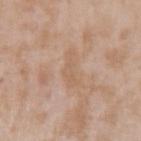Q: Was this lesion biopsied?
A: no biopsy performed (imaged during a skin exam)
Q: Patient demographics?
A: male, about 45 years old
Q: What is the imaging modality?
A: ~15 mm crop, total-body skin-cancer survey
Q: How large is the lesion?
A: ≈4.5 mm
Q: Where on the body is the lesion?
A: the left upper arm
Q: What did automated image analysis measure?
A: a lesion area of about 4.5 mm², a shape eccentricity near 0.95, and two-axis asymmetry of about 0.4; a border-irregularity index near 6/10, internal color variation of about 0.5 on a 0–10 scale, and peripheral color asymmetry of about 0
Q: Illumination type?
A: white-light illumination